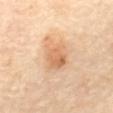Case summary:
* workup — no biopsy performed (imaged during a skin exam)
* patient — female, about 65 years old
* image — total-body-photography crop, ~15 mm field of view
* anatomic site — the mid back
* image-analysis metrics — a nevus-likeness score of about 75/100 and lesion-presence confidence of about 100/100
* size — ~5 mm (longest diameter)
* illumination — cross-polarized